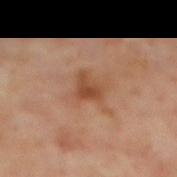<record>
<biopsy_status>not biopsied; imaged during a skin examination</biopsy_status>
<lesion_size>
  <long_diameter_mm_approx>3.0</long_diameter_mm_approx>
</lesion_size>
<site>mid back</site>
<lighting>cross-polarized</lighting>
<patient>
  <sex>male</sex>
  <age_approx>65</age_approx>
</patient>
<image>
  <source>total-body photography crop</source>
  <field_of_view_mm>15</field_of_view_mm>
</image>
</record>